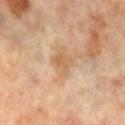notes=imaged on a skin check; not biopsied | imaging modality=~15 mm tile from a whole-body skin photo | automated lesion analysis=a footprint of about 6 mm² and two-axis asymmetry of about 0.3; border irregularity of about 3.5 on a 0–10 scale, internal color variation of about 2.5 on a 0–10 scale, and radial color variation of about 1; a detector confidence of about 100 out of 100 that the crop contains a lesion | size=≈3 mm | lighting=cross-polarized illumination | subject=female, about 65 years old | body site=the left leg.